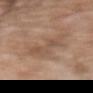follow-up: catalogued during a skin exam; not biopsied | location: the right upper arm | image: 15 mm crop, total-body photography | patient: female, aged 73 to 77.